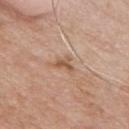Captured under white-light illumination. The recorded lesion diameter is about 2.5 mm. On the front of the torso. An algorithmic analysis of the crop reported a border-irregularity index near 5.5/10, internal color variation of about 1.5 on a 0–10 scale, and radial color variation of about 0.5. And it measured lesion-presence confidence of about 100/100. A male subject in their mid- to late 50s. A close-up tile cropped from a whole-body skin photograph, about 15 mm across.